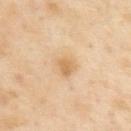workup: catalogued during a skin exam; not biopsied | image source: ~15 mm crop, total-body skin-cancer survey | patient: male, aged approximately 55 | anatomic site: the back | lesion diameter: ~4 mm (longest diameter) | tile lighting: cross-polarized.